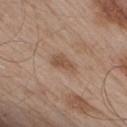body site=the right upper arm
imaging modality=total-body-photography crop, ~15 mm field of view
lighting=white-light
size=~3.5 mm (longest diameter)
subject=male, aged around 55